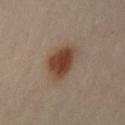Clinical impression: Part of a total-body skin-imaging series; this lesion was reviewed on a skin check and was not flagged for biopsy. Background: The patient is a female aged approximately 40. Automated tile analysis of the lesion measured about 12 CIELAB-L* units darker than the surrounding skin and a normalized lesion–skin contrast near 10. The analysis additionally found a border-irregularity index near 2.5/10 and a peripheral color-asymmetry measure near 1. The tile uses cross-polarized illumination. A lesion tile, about 15 mm wide, cut from a 3D total-body photograph. The lesion is on the left arm. Approximately 4.5 mm at its widest.